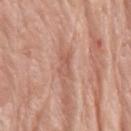The lesion was photographed on a routine skin check and not biopsied; there is no pathology result.
A female patient, roughly 75 years of age.
A 15 mm crop from a total-body photograph taken for skin-cancer surveillance.
On the right upper arm.